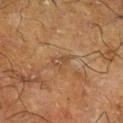Clinical impression:
Imaged during a routine full-body skin examination; the lesion was not biopsied and no histopathology is available.
Context:
Approximately 2.5 mm at its widest. A region of skin cropped from a whole-body photographic capture, roughly 15 mm wide. A male subject in their mid-60s. The lesion is on the leg. The tile uses cross-polarized illumination.